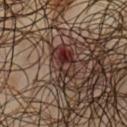* workup · catalogued during a skin exam; not biopsied
* anatomic site · the front of the torso
* patient · male, approximately 65 years of age
* image · ~15 mm crop, total-body skin-cancer survey
* tile lighting · cross-polarized illumination
* size · about 5 mm